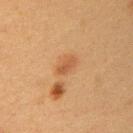Clinical impression:
Imaged during a routine full-body skin examination; the lesion was not biopsied and no histopathology is available.
Image and clinical context:
A female patient aged 38 to 42. A roughly 15 mm field-of-view crop from a total-body skin photograph. On the left upper arm.